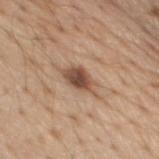Impression:
This lesion was catalogued during total-body skin photography and was not selected for biopsy.
Acquisition and patient details:
The lesion-visualizer software estimated two-axis asymmetry of about 0.25. The analysis additionally found a lesion color around L≈50 a*≈19 b*≈29 in CIELAB and about 14 CIELAB-L* units darker than the surrounding skin. It also reported border irregularity of about 3 on a 0–10 scale, internal color variation of about 4 on a 0–10 scale, and a peripheral color-asymmetry measure near 1. Cropped from a whole-body photographic skin survey; the tile spans about 15 mm. A male patient, aged 68–72. The lesion is located on the front of the torso. Captured under white-light illumination. The lesion's longest dimension is about 4 mm.